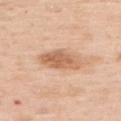<lesion>
<biopsy_status>not biopsied; imaged during a skin examination</biopsy_status>
<patient>
  <sex>female</sex>
  <age_approx>50</age_approx>
</patient>
<site>upper back</site>
<image>
  <source>total-body photography crop</source>
  <field_of_view_mm>15</field_of_view_mm>
</image>
</lesion>